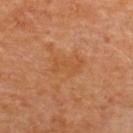{"biopsy_status": "not biopsied; imaged during a skin examination", "image": {"source": "total-body photography crop", "field_of_view_mm": 15}, "patient": {"sex": "female", "age_approx": 50}, "lesion_size": {"long_diameter_mm_approx": 3.5}, "site": "back", "lighting": "cross-polarized"}